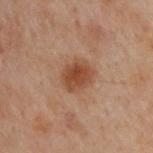– biopsy status: total-body-photography surveillance lesion; no biopsy
– lighting: cross-polarized illumination
– subject: male, aged 48–52
– imaging modality: ~15 mm tile from a whole-body skin photo
– lesion size: ~3.5 mm (longest diameter)
– TBP lesion metrics: an area of roughly 8 mm², an outline eccentricity of about 0.6 (0 = round, 1 = elongated), and two-axis asymmetry of about 0.15; an average lesion color of about L≈37 a*≈19 b*≈27 (CIELAB), about 9 CIELAB-L* units darker than the surrounding skin, and a normalized lesion–skin contrast near 8.5
– anatomic site: the upper back